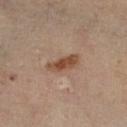Captured during whole-body skin photography for melanoma surveillance; the lesion was not biopsied.
The recorded lesion diameter is about 4 mm.
Imaged with cross-polarized lighting.
The lesion is located on the right lower leg.
A female patient, approximately 60 years of age.
A lesion tile, about 15 mm wide, cut from a 3D total-body photograph.